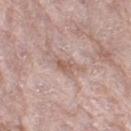{
  "biopsy_status": "not biopsied; imaged during a skin examination",
  "site": "right thigh",
  "patient": {
    "sex": "female",
    "age_approx": 70
  },
  "image": {
    "source": "total-body photography crop",
    "field_of_view_mm": 15
  },
  "lesion_size": {
    "long_diameter_mm_approx": 3.0
  }
}